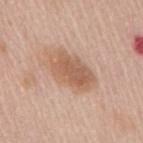Recorded during total-body skin imaging; not selected for excision or biopsy. Cropped from a total-body skin-imaging series; the visible field is about 15 mm. About 6 mm across. The subject is a female aged 63–67. From the mid back. An algorithmic analysis of the crop reported a footprint of about 14 mm², an outline eccentricity of about 0.85 (0 = round, 1 = elongated), and two-axis asymmetry of about 0.15. It also reported a lesion color around L≈59 a*≈20 b*≈31 in CIELAB, about 10 CIELAB-L* units darker than the surrounding skin, and a normalized lesion–skin contrast near 7. It also reported internal color variation of about 3.5 on a 0–10 scale.